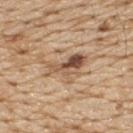Impression:
The lesion was tiled from a total-body skin photograph and was not biopsied.
Acquisition and patient details:
A 15 mm close-up tile from a total-body photography series done for melanoma screening. Imaged with white-light lighting. The lesion is on the back. Longest diameter approximately 6 mm. The patient is a male aged 68 to 72. Automated tile analysis of the lesion measured a footprint of about 11 mm², an outline eccentricity of about 0.85 (0 = round, 1 = elongated), and two-axis asymmetry of about 0.5. It also reported a mean CIELAB color near L≈55 a*≈18 b*≈32, a lesion–skin lightness drop of about 12, and a normalized lesion–skin contrast near 8. And it measured internal color variation of about 10 on a 0–10 scale and peripheral color asymmetry of about 4.